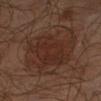follow-up: imaged on a skin check; not biopsied | TBP lesion metrics: about 6 CIELAB-L* units darker than the surrounding skin and a lesion-to-skin contrast of about 6.5 (normalized; higher = more distinct) | image: 15 mm crop, total-body photography | location: the right upper arm | subject: male, aged around 65 | illumination: cross-polarized illumination | diameter: ~6.5 mm (longest diameter).